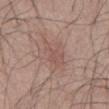Q: Was this lesion biopsied?
A: total-body-photography surveillance lesion; no biopsy
Q: How was the tile lit?
A: white-light
Q: Where on the body is the lesion?
A: the abdomen
Q: What are the patient's age and sex?
A: male, aged 53–57
Q: What is the lesion's diameter?
A: about 5 mm
Q: What is the imaging modality?
A: ~15 mm crop, total-body skin-cancer survey